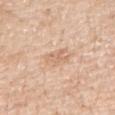Q: Was a biopsy performed?
A: catalogued during a skin exam; not biopsied
Q: What is the imaging modality?
A: 15 mm crop, total-body photography
Q: How large is the lesion?
A: ~2.5 mm (longest diameter)
Q: What are the patient's age and sex?
A: female, aged 43–47
Q: Lesion location?
A: the right upper arm
Q: What did automated image analysis measure?
A: a footprint of about 5 mm², an eccentricity of roughly 0.5, and a shape-asymmetry score of about 0.4 (0 = symmetric); a border-irregularity index near 4.5/10, a within-lesion color-variation index near 2/10, and peripheral color asymmetry of about 0.5
Q: How was the tile lit?
A: white-light illumination